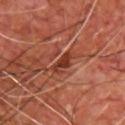| field | value |
|---|---|
| biopsy status | imaged on a skin check; not biopsied |
| patient | male, about 65 years old |
| lesion size | ~3 mm (longest diameter) |
| image-analysis metrics | an area of roughly 3.5 mm², a shape eccentricity near 0.9, and a shape-asymmetry score of about 0.3 (0 = symmetric); a detector confidence of about 100 out of 100 that the crop contains a lesion |
| location | the chest |
| acquisition | ~15 mm tile from a whole-body skin photo |